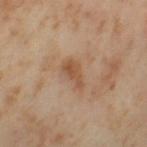Recorded during total-body skin imaging; not selected for excision or biopsy. A female subject roughly 55 years of age. The lesion is on the left thigh. A 15 mm close-up tile from a total-body photography series done for melanoma screening. This is a cross-polarized tile.